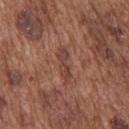Imaged during a routine full-body skin examination; the lesion was not biopsied and no histopathology is available.
A male subject, roughly 75 years of age.
The recorded lesion diameter is about 4 mm.
A lesion tile, about 15 mm wide, cut from a 3D total-body photograph.
On the back.
The lesion-visualizer software estimated a lesion color around L≈42 a*≈22 b*≈25 in CIELAB, roughly 8 lightness units darker than nearby skin, and a normalized border contrast of about 6.5. The analysis additionally found an automated nevus-likeness rating near 0 out of 100.
Captured under white-light illumination.